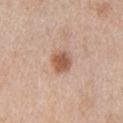Case summary:
- size: ≈2.5 mm
- patient: female, aged 63 to 67
- body site: the right upper arm
- illumination: white-light illumination
- imaging modality: total-body-photography crop, ~15 mm field of view